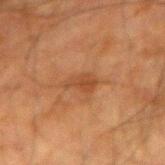Notes:
- workup: total-body-photography surveillance lesion; no biopsy
- TBP lesion metrics: a lesion area of about 4.5 mm², a shape eccentricity near 0.8, and a symmetry-axis asymmetry near 0.45; an average lesion color of about L≈37 a*≈20 b*≈31 (CIELAB) and a lesion-to-skin contrast of about 6 (normalized; higher = more distinct); a border-irregularity index near 4/10 and peripheral color asymmetry of about 0.5
- lighting: cross-polarized
- patient: male, about 80 years old
- imaging modality: total-body-photography crop, ~15 mm field of view
- lesion diameter: about 3.5 mm
- site: the right forearm